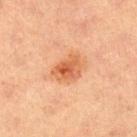Recorded during total-body skin imaging; not selected for excision or biopsy.